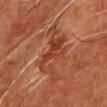• biopsy status · no biopsy performed (imaged during a skin exam)